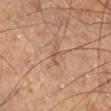Assessment: The lesion was photographed on a routine skin check and not biopsied; there is no pathology result. Context: The patient is a male aged 48 to 52. A roughly 15 mm field-of-view crop from a total-body skin photograph. About 3 mm across. From the right lower leg. This is a cross-polarized tile.